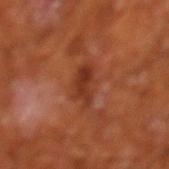<case>
<biopsy_status>not biopsied; imaged during a skin examination</biopsy_status>
<site>leg</site>
<image>
  <source>total-body photography crop</source>
  <field_of_view_mm>15</field_of_view_mm>
</image>
<patient>
  <sex>male</sex>
  <age_approx>65</age_approx>
</patient>
<automated_metrics>
  <cielab_L>34</cielab_L>
  <cielab_a>27</cielab_a>
  <cielab_b>31</cielab_b>
  <vs_skin_darker_L>9.0</vs_skin_darker_L>
  <vs_skin_contrast_norm>7.5</vs_skin_contrast_norm>
  <color_variation_0_10>3.5</color_variation_0_10>
  <peripheral_color_asymmetry>1.5</peripheral_color_asymmetry>
  <nevus_likeness_0_100>15</nevus_likeness_0_100>
</automated_metrics>
<lighting>cross-polarized</lighting>
<lesion_size>
  <long_diameter_mm_approx>3.5</long_diameter_mm_approx>
</lesion_size>
</case>